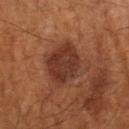Recorded during total-body skin imaging; not selected for excision or biopsy. A lesion tile, about 15 mm wide, cut from a 3D total-body photograph. A female subject about 80 years old. The lesion is on the arm.